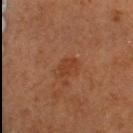{
  "biopsy_status": "not biopsied; imaged during a skin examination",
  "patient": {
    "sex": "female",
    "age_approx": 70
  },
  "image": {
    "source": "total-body photography crop",
    "field_of_view_mm": 15
  },
  "lesion_size": {
    "long_diameter_mm_approx": 2.5
  },
  "site": "head or neck",
  "lighting": "cross-polarized"
}